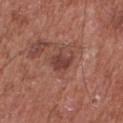The lesion was tiled from a total-body skin photograph and was not biopsied.
Located on the front of the torso.
A male patient about 75 years old.
The tile uses white-light illumination.
Measured at roughly 3 mm in maximum diameter.
Cropped from a whole-body photographic skin survey; the tile spans about 15 mm.